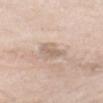workup = catalogued during a skin exam; not biopsied | patient = male, in their mid-60s | imaging modality = total-body-photography crop, ~15 mm field of view | site = the back.